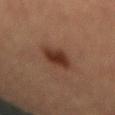Notes:
– workup — no biopsy performed (imaged during a skin exam)
– TBP lesion metrics — a footprint of about 7.5 mm², a shape eccentricity near 0.7, and a symmetry-axis asymmetry near 0.2
– lighting — cross-polarized illumination
– body site — the mid back
– subject — female, roughly 70 years of age
– acquisition — ~15 mm crop, total-body skin-cancer survey
– lesion diameter — ≈3.5 mm